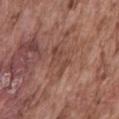Q: Is there a histopathology result?
A: total-body-photography surveillance lesion; no biopsy
Q: What did automated image analysis measure?
A: an area of roughly 5.5 mm² and a symmetry-axis asymmetry near 0.55; a mean CIELAB color near L≈45 a*≈21 b*≈26, a lesion–skin lightness drop of about 7, and a normalized border contrast of about 5.5
Q: Where on the body is the lesion?
A: the mid back
Q: What is the imaging modality?
A: ~15 mm tile from a whole-body skin photo
Q: What lighting was used for the tile?
A: white-light illumination
Q: What are the patient's age and sex?
A: male, roughly 75 years of age